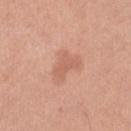biopsy status: imaged on a skin check; not biopsied | location: the leg | diameter: ≈3 mm | patient: female, aged 53 to 57 | illumination: white-light illumination | imaging modality: ~15 mm crop, total-body skin-cancer survey | image-analysis metrics: a mean CIELAB color near L≈61 a*≈24 b*≈31, about 7 CIELAB-L* units darker than the surrounding skin, and a lesion-to-skin contrast of about 5 (normalized; higher = more distinct).